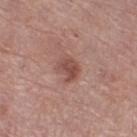Impression:
Recorded during total-body skin imaging; not selected for excision or biopsy.
Background:
The recorded lesion diameter is about 3.5 mm. The patient is a female roughly 65 years of age. Automated image analysis of the tile measured a border-irregularity index near 3/10, a within-lesion color-variation index near 3.5/10, and peripheral color asymmetry of about 1.5. On the leg. Captured under white-light illumination. A 15 mm close-up extracted from a 3D total-body photography capture.